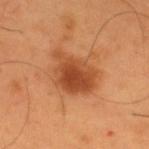Impression: Part of a total-body skin-imaging series; this lesion was reviewed on a skin check and was not flagged for biopsy. Acquisition and patient details: The lesion is on the upper back. A male subject, roughly 55 years of age. Approximately 5.5 mm at its widest. This is a cross-polarized tile. The total-body-photography lesion software estimated an area of roughly 16 mm². A roughly 15 mm field-of-view crop from a total-body skin photograph.